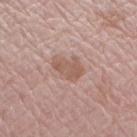Assessment: Recorded during total-body skin imaging; not selected for excision or biopsy. Clinical summary: On the right forearm. The patient is a male roughly 70 years of age. Cropped from a total-body skin-imaging series; the visible field is about 15 mm. Measured at roughly 3.5 mm in maximum diameter. The lesion-visualizer software estimated an automated nevus-likeness rating near 30 out of 100 and a detector confidence of about 100 out of 100 that the crop contains a lesion.